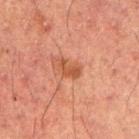The lesion was photographed on a routine skin check and not biopsied; there is no pathology result.